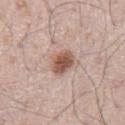This lesion was catalogued during total-body skin photography and was not selected for biopsy. A roughly 15 mm field-of-view crop from a total-body skin photograph. Automated tile analysis of the lesion measured an automated nevus-likeness rating near 95 out of 100 and lesion-presence confidence of about 100/100. The lesion's longest dimension is about 3.5 mm. From the abdomen. Captured under white-light illumination. A male subject in their mid-60s.